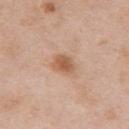Notes:
• workup · imaged on a skin check; not biopsied